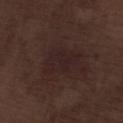Measured at roughly 4.5 mm in maximum diameter. The tile uses white-light illumination. The patient is a male aged 68 to 72. Located on the left lower leg. Automated image analysis of the tile measured a lesion area of about 9.5 mm², an eccentricity of roughly 0.75, and a symmetry-axis asymmetry near 0.35. It also reported a border-irregularity rating of about 4.5/10, a within-lesion color-variation index near 2/10, and radial color variation of about 0.5. A close-up tile cropped from a whole-body skin photograph, about 15 mm across.